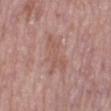Imaged during a routine full-body skin examination; the lesion was not biopsied and no histopathology is available.
A 15 mm close-up extracted from a 3D total-body photography capture.
About 5 mm across.
A female subject roughly 60 years of age.
The tile uses white-light illumination.
Located on the leg.